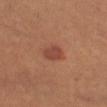Q: Was this lesion biopsied?
A: total-body-photography surveillance lesion; no biopsy
Q: Who is the patient?
A: male, aged approximately 55
Q: Where on the body is the lesion?
A: the left thigh
Q: Illumination type?
A: cross-polarized illumination
Q: What is the imaging modality?
A: 15 mm crop, total-body photography
Q: How large is the lesion?
A: ≈3 mm